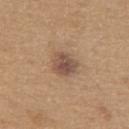Imaged during a routine full-body skin examination; the lesion was not biopsied and no histopathology is available.
On the upper back.
A male patient, about 65 years old.
A 15 mm crop from a total-body photograph taken for skin-cancer surveillance.